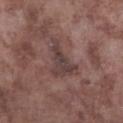{
  "site": "leg",
  "patient": {
    "sex": "male",
    "age_approx": 75
  },
  "image": {
    "source": "total-body photography crop",
    "field_of_view_mm": 15
  }
}